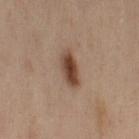Q: Is there a histopathology result?
A: total-body-photography surveillance lesion; no biopsy
Q: Lesion size?
A: ~4 mm (longest diameter)
Q: What is the imaging modality?
A: total-body-photography crop, ~15 mm field of view
Q: Illumination type?
A: cross-polarized illumination
Q: What is the anatomic site?
A: the back
Q: Who is the patient?
A: female, approximately 55 years of age